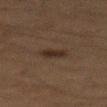Captured during whole-body skin photography for melanoma surveillance; the lesion was not biopsied.
The tile uses cross-polarized illumination.
From the mid back.
A male subject aged approximately 65.
A lesion tile, about 15 mm wide, cut from a 3D total-body photograph.